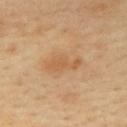Findings:
• notes · no biopsy performed (imaged during a skin exam)
• lighting · cross-polarized illumination
• TBP lesion metrics · an outline eccentricity of about 0.9 (0 = round, 1 = elongated) and a symmetry-axis asymmetry near 0.45; a border-irregularity rating of about 6/10, a within-lesion color-variation index near 1/10, and radial color variation of about 0.5
• subject · female, aged approximately 45
• image · 15 mm crop, total-body photography
• location · the upper back